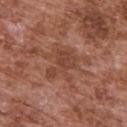Part of a total-body skin-imaging series; this lesion was reviewed on a skin check and was not flagged for biopsy. A male patient roughly 75 years of age. A close-up tile cropped from a whole-body skin photograph, about 15 mm across. An algorithmic analysis of the crop reported a border-irregularity index near 7.5/10, a color-variation rating of about 2.5/10, and radial color variation of about 0.5. The analysis additionally found an automated nevus-likeness rating near 0 out of 100 and a lesion-detection confidence of about 100/100. Approximately 4.5 mm at its widest. The lesion is located on the upper back. Captured under white-light illumination.